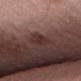follow-up: imaged on a skin check; not biopsied
illumination: white-light
anatomic site: the back
size: ≈3 mm
patient: male, about 50 years old
image: 15 mm crop, total-body photography